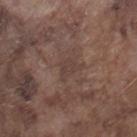follow-up: total-body-photography surveillance lesion; no biopsy